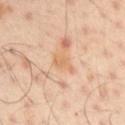Clinical impression: Captured during whole-body skin photography for melanoma surveillance; the lesion was not biopsied. Clinical summary: Automated tile analysis of the lesion measured a lesion area of about 4 mm², an outline eccentricity of about 0.8 (0 = round, 1 = elongated), and a shape-asymmetry score of about 0.35 (0 = symmetric). The software also gave a mean CIELAB color near L≈67 a*≈20 b*≈37, roughly 7 lightness units darker than nearby skin, and a lesion-to-skin contrast of about 6 (normalized; higher = more distinct). A male patient, aged around 50. Located on the left arm. This image is a 15 mm lesion crop taken from a total-body photograph. The lesion's longest dimension is about 3 mm. This is a cross-polarized tile.